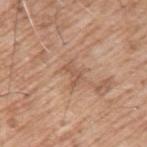The lesion is on the left upper arm. A lesion tile, about 15 mm wide, cut from a 3D total-body photograph. The lesion's longest dimension is about 2.5 mm. A male subject aged approximately 60.